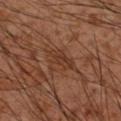- notes — total-body-photography surveillance lesion; no biopsy